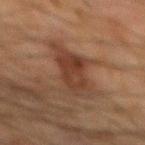The lesion's longest dimension is about 7 mm.
The lesion is located on the mid back.
A roughly 15 mm field-of-view crop from a total-body skin photograph.
A male patient approximately 65 years of age.
Captured under cross-polarized illumination.